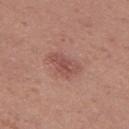Assessment:
The lesion was photographed on a routine skin check and not biopsied; there is no pathology result.
Context:
The lesion's longest dimension is about 3.5 mm. On the leg. This is a white-light tile. A close-up tile cropped from a whole-body skin photograph, about 15 mm across. The patient is a female aged 28 to 32.